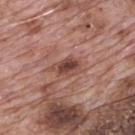Case summary:
* biopsy status — catalogued during a skin exam; not biopsied
* tile lighting — white-light illumination
* automated metrics — a footprint of about 6.5 mm² and an outline eccentricity of about 0.8 (0 = round, 1 = elongated); a mean CIELAB color near L≈45 a*≈23 b*≈25 and a lesion-to-skin contrast of about 8.5 (normalized; higher = more distinct); a border-irregularity rating of about 3.5/10
* acquisition — total-body-photography crop, ~15 mm field of view
* patient — male, aged around 70
* size — ≈4 mm
* anatomic site — the mid back